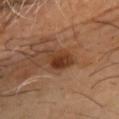Assessment: Captured during whole-body skin photography for melanoma surveillance; the lesion was not biopsied. Acquisition and patient details: Captured under cross-polarized illumination. A male subject about 65 years old. Approximately 4 mm at its widest. A region of skin cropped from a whole-body photographic capture, roughly 15 mm wide. From the chest.